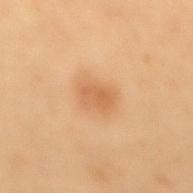Clinical impression: Recorded during total-body skin imaging; not selected for excision or biopsy. Acquisition and patient details: Imaged with cross-polarized lighting. Approximately 3 mm at its widest. The lesion is on the mid back. Cropped from a whole-body photographic skin survey; the tile spans about 15 mm. A male patient, aged 53–57. Automated tile analysis of the lesion measured an outline eccentricity of about 0.7 (0 = round, 1 = elongated) and two-axis asymmetry of about 0.2. The analysis additionally found a lesion color around L≈50 a*≈19 b*≈34 in CIELAB, roughly 7 lightness units darker than nearby skin, and a normalized border contrast of about 5.5.